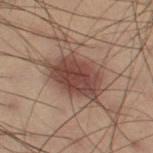Impression:
Imaged during a routine full-body skin examination; the lesion was not biopsied and no histopathology is available.
Context:
Imaged with cross-polarized lighting. This image is a 15 mm lesion crop taken from a total-body photograph. The lesion's longest dimension is about 6.5 mm. The subject is a male in their mid-50s. The lesion is on the right thigh.